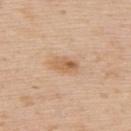Case summary:
- notes — catalogued during a skin exam; not biopsied
- lighting — white-light
- subject — male, approximately 60 years of age
- image — ~15 mm crop, total-body skin-cancer survey
- lesion diameter — ≈3 mm
- location — the back